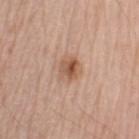| field | value |
|---|---|
| imaging modality | total-body-photography crop, ~15 mm field of view |
| site | the left upper arm |
| patient | male, in their mid- to late 60s |